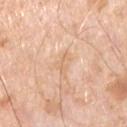| field | value |
|---|---|
| biopsy status | imaged on a skin check; not biopsied |
| site | the chest |
| subject | male, aged 68 to 72 |
| acquisition | ~15 mm tile from a whole-body skin photo |